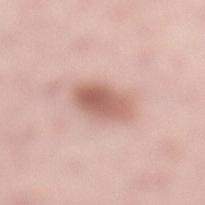Imaged during a routine full-body skin examination; the lesion was not biopsied and no histopathology is available.